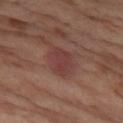notes: no biopsy performed (imaged during a skin exam); illumination: cross-polarized; size: about 3.5 mm; subject: female, in their mid-50s; anatomic site: the left thigh; acquisition: 15 mm crop, total-body photography.